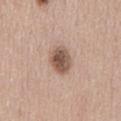follow-up — total-body-photography surveillance lesion; no biopsy | acquisition — ~15 mm tile from a whole-body skin photo | anatomic site — the abdomen | patient — male, aged approximately 60.